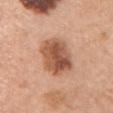Impression:
The lesion was photographed on a routine skin check and not biopsied; there is no pathology result.
Clinical summary:
This is a white-light tile. This image is a 15 mm lesion crop taken from a total-body photograph. Automated image analysis of the tile measured a lesion area of about 16 mm² and a shape eccentricity near 0.7. The analysis additionally found a lesion color around L≈55 a*≈23 b*≈33 in CIELAB, about 14 CIELAB-L* units darker than the surrounding skin, and a normalized lesion–skin contrast near 9.5. The software also gave border irregularity of about 2 on a 0–10 scale, internal color variation of about 6.5 on a 0–10 scale, and peripheral color asymmetry of about 2. And it measured a lesion-detection confidence of about 100/100. The lesion is on the left upper arm. A female patient in their 60s. Longest diameter approximately 5 mm.